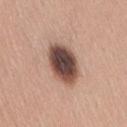biopsy status: catalogued during a skin exam; not biopsied | patient: female, aged approximately 30 | image-analysis metrics: a shape eccentricity near 0.7; a mean CIELAB color near L≈46 a*≈19 b*≈23, roughly 21 lightness units darker than nearby skin, and a lesion-to-skin contrast of about 14 (normalized; higher = more distinct); a detector confidence of about 100 out of 100 that the crop contains a lesion | body site: the back | image source: ~15 mm tile from a whole-body skin photo | size: ~5 mm (longest diameter).